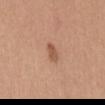Captured during whole-body skin photography for melanoma surveillance; the lesion was not biopsied. A lesion tile, about 15 mm wide, cut from a 3D total-body photograph. Captured under white-light illumination. The lesion's longest dimension is about 2.5 mm. A female patient aged approximately 35. From the mid back.